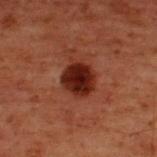image: total-body-photography crop, ~15 mm field of view | size: ≈3.5 mm | automated lesion analysis: an area of roughly 10 mm², an eccentricity of roughly 0.35, and two-axis asymmetry of about 0.15; a mean CIELAB color near L≈19 a*≈22 b*≈22, roughly 12 lightness units darker than nearby skin, and a normalized lesion–skin contrast near 13; a classifier nevus-likeness of about 90/100 and a lesion-detection confidence of about 100/100 | illumination: cross-polarized illumination | location: the upper back | patient: male, aged 58 to 62.